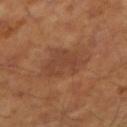Q: Was a biopsy performed?
A: total-body-photography surveillance lesion; no biopsy
Q: What is the anatomic site?
A: the right forearm
Q: Patient demographics?
A: male, in their mid-40s
Q: Illumination type?
A: cross-polarized illumination
Q: How was this image acquired?
A: total-body-photography crop, ~15 mm field of view
Q: How large is the lesion?
A: about 5.5 mm
Q: Automated lesion metrics?
A: an area of roughly 16 mm², a shape eccentricity near 0.6, and a symmetry-axis asymmetry near 0.2; a normalized lesion–skin contrast near 5; border irregularity of about 3 on a 0–10 scale, a within-lesion color-variation index near 2.5/10, and a peripheral color-asymmetry measure near 1